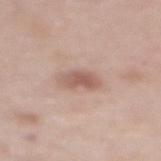Clinical impression:
Recorded during total-body skin imaging; not selected for excision or biopsy.
Background:
The patient is a female approximately 65 years of age. Located on the upper back. Captured under white-light illumination. A 15 mm close-up extracted from a 3D total-body photography capture. An algorithmic analysis of the crop reported an eccentricity of roughly 0.85 and a shape-asymmetry score of about 0.25 (0 = symmetric). The analysis additionally found a border-irregularity rating of about 3/10 and internal color variation of about 4 on a 0–10 scale. It also reported a nevus-likeness score of about 40/100 and a lesion-detection confidence of about 100/100. The lesion's longest dimension is about 3.5 mm.